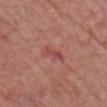  biopsy_status: not biopsied; imaged during a skin examination
  lesion_size:
    long_diameter_mm_approx: 3.0
  lighting: white-light
  site: head or neck
  image:
    source: total-body photography crop
    field_of_view_mm: 15
  automated_metrics:
    eccentricity: 0.9
    shape_asymmetry: 0.3
    cielab_L: 48
    cielab_a: 29
    cielab_b: 23
    vs_skin_darker_L: 8.0
    vs_skin_contrast_norm: 6.0
    border_irregularity_0_10: 4.0
    color_variation_0_10: 0.5
    peripheral_color_asymmetry: 0.0
  patient:
    sex: male
    age_approx: 65